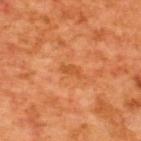The lesion was photographed on a routine skin check and not biopsied; there is no pathology result. The subject is a male about 65 years old. Located on the upper back. Cropped from a whole-body photographic skin survey; the tile spans about 15 mm. Approximately 2.5 mm at its widest. The lesion-visualizer software estimated an area of roughly 2.5 mm² and an eccentricity of roughly 0.85. The analysis additionally found a lesion color around L≈52 a*≈30 b*≈45 in CIELAB, a lesion–skin lightness drop of about 7, and a normalized lesion–skin contrast near 6. And it measured a border-irregularity index near 4/10, a color-variation rating of about 0.5/10, and peripheral color asymmetry of about 0.